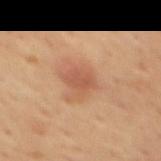Image and clinical context:
Measured at roughly 3 mm in maximum diameter. An algorithmic analysis of the crop reported a within-lesion color-variation index near 1/10 and peripheral color asymmetry of about 0.5. The subject is a male aged around 65. A close-up tile cropped from a whole-body skin photograph, about 15 mm across. Located on the mid back.